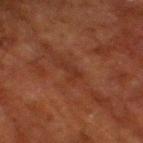workup: no biopsy performed (imaged during a skin exam) | diameter: about 3 mm | subject: male, aged around 80 | imaging modality: total-body-photography crop, ~15 mm field of view | tile lighting: cross-polarized illumination | location: the right thigh | TBP lesion metrics: a mean CIELAB color near L≈25 a*≈21 b*≈25, about 4 CIELAB-L* units darker than the surrounding skin, and a lesion-to-skin contrast of about 5 (normalized; higher = more distinct); a nevus-likeness score of about 0/100 and a detector confidence of about 85 out of 100 that the crop contains a lesion.